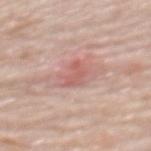Clinical impression:
No biopsy was performed on this lesion — it was imaged during a full skin examination and was not determined to be concerning.
Clinical summary:
On the upper back. A 15 mm crop from a total-body photograph taken for skin-cancer surveillance. Automated image analysis of the tile measured an area of roughly 4.5 mm², an eccentricity of roughly 0.75, and a shape-asymmetry score of about 0.45 (0 = symmetric). The software also gave border irregularity of about 4.5 on a 0–10 scale, internal color variation of about 1.5 on a 0–10 scale, and a peripheral color-asymmetry measure near 0.5. The analysis additionally found a nevus-likeness score of about 0/100 and a detector confidence of about 100 out of 100 that the crop contains a lesion. A female patient, aged 63 to 67. Captured under white-light illumination. The recorded lesion diameter is about 2.5 mm.